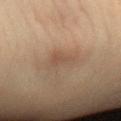{
  "biopsy_status": "not biopsied; imaged during a skin examination",
  "image": {
    "source": "total-body photography crop",
    "field_of_view_mm": 15
  },
  "site": "left forearm",
  "lighting": "cross-polarized",
  "patient": {
    "sex": "female",
    "age_approx": 30
  }
}